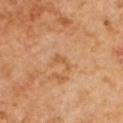The lesion was tiled from a total-body skin photograph and was not biopsied. Captured under cross-polarized illumination. A male subject roughly 65 years of age. A region of skin cropped from a whole-body photographic capture, roughly 15 mm wide.